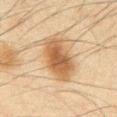biopsy_status: not biopsied; imaged during a skin examination
automated_metrics:
  area_mm2_approx: 16.0
  eccentricity: 0.8
  shape_asymmetry: 0.15
site: abdomen
lighting: cross-polarized
patient:
  sex: male
  age_approx: 65
image:
  source: total-body photography crop
  field_of_view_mm: 15